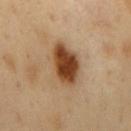Clinical impression:
Captured during whole-body skin photography for melanoma surveillance; the lesion was not biopsied.
Context:
A male subject about 50 years old. A lesion tile, about 15 mm wide, cut from a 3D total-body photograph. Captured under cross-polarized illumination. The lesion is located on the back.